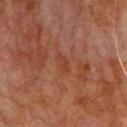<lesion>
<biopsy_status>not biopsied; imaged during a skin examination</biopsy_status>
<image>
  <source>total-body photography crop</source>
  <field_of_view_mm>15</field_of_view_mm>
</image>
<patient>
  <sex>male</sex>
  <age_approx>80</age_approx>
</patient>
<site>chest</site>
</lesion>